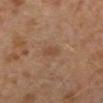The lesion was photographed on a routine skin check and not biopsied; there is no pathology result.
A male subject about 30 years old.
This image is a 15 mm lesion crop taken from a total-body photograph.
From the left lower leg.